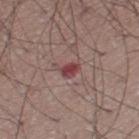Q: Is there a histopathology result?
A: catalogued during a skin exam; not biopsied
Q: Who is the patient?
A: male, aged 53–57
Q: What kind of image is this?
A: total-body-photography crop, ~15 mm field of view